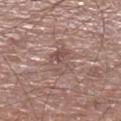image = ~15 mm crop, total-body skin-cancer survey; patient = male, aged 68–72; lighting = white-light; lesion size = ~3.5 mm (longest diameter); anatomic site = the left lower leg.